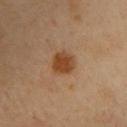Clinical impression:
The lesion was photographed on a routine skin check and not biopsied; there is no pathology result.
Context:
The tile uses cross-polarized illumination. The lesion is located on the chest. Automated image analysis of the tile measured a lesion color around L≈44 a*≈22 b*≈36 in CIELAB, a lesion–skin lightness drop of about 11, and a normalized border contrast of about 9.5. The analysis additionally found a nevus-likeness score of about 100/100 and a detector confidence of about 100 out of 100 that the crop contains a lesion. A 15 mm close-up extracted from a 3D total-body photography capture. The subject is a male approximately 40 years of age. Longest diameter approximately 2.5 mm.